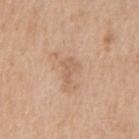Q: Was a biopsy performed?
A: total-body-photography surveillance lesion; no biopsy
Q: Lesion location?
A: the chest
Q: Lesion size?
A: about 3 mm
Q: What are the patient's age and sex?
A: male, aged 68 to 72
Q: Automated lesion metrics?
A: a footprint of about 4 mm², an eccentricity of roughly 0.85, and a shape-asymmetry score of about 0.45 (0 = symmetric); a mean CIELAB color near L≈61 a*≈18 b*≈32 and a lesion–skin lightness drop of about 7; a border-irregularity index near 5/10, a within-lesion color-variation index near 1/10, and radial color variation of about 0.5; an automated nevus-likeness rating near 0 out of 100 and a lesion-detection confidence of about 100/100
Q: How was this image acquired?
A: ~15 mm tile from a whole-body skin photo